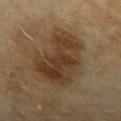biopsy status = total-body-photography surveillance lesion; no biopsy | site = the right forearm | image = 15 mm crop, total-body photography | lighting = cross-polarized | patient = female, aged 58 to 62 | size = ≈8.5 mm.